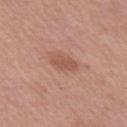Q: Was this lesion biopsied?
A: catalogued during a skin exam; not biopsied